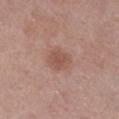  biopsy_status: not biopsied; imaged during a skin examination
  lighting: white-light
  lesion_size:
    long_diameter_mm_approx: 3.0
  patient:
    sex: female
    age_approx: 50
  site: right lower leg
  image:
    source: total-body photography crop
    field_of_view_mm: 15